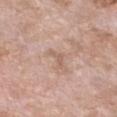Part of a total-body skin-imaging series; this lesion was reviewed on a skin check and was not flagged for biopsy. The lesion is located on the chest. This image is a 15 mm lesion crop taken from a total-body photograph. The patient is a female aged approximately 75.